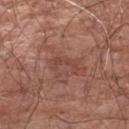Findings:
* biopsy status · total-body-photography surveillance lesion; no biopsy
* diameter · about 3.5 mm
* lighting · white-light illumination
* subject · male, aged 63–67
* site · the left upper arm
* acquisition · ~15 mm tile from a whole-body skin photo